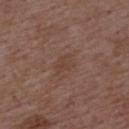workup: catalogued during a skin exam; not biopsied
acquisition: total-body-photography crop, ~15 mm field of view
lesion diameter: about 2.5 mm
lighting: white-light illumination
patient: male, in their 50s
image-analysis metrics: an outline eccentricity of about 0.75 (0 = round, 1 = elongated) and a symmetry-axis asymmetry near 0.4; an average lesion color of about L≈41 a*≈18 b*≈25 (CIELAB) and roughly 5 lightness units darker than nearby skin; a border-irregularity index near 4.5/10, a color-variation rating of about 1/10, and radial color variation of about 0
body site: the upper back